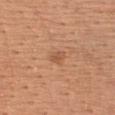Imaged during a routine full-body skin examination; the lesion was not biopsied and no histopathology is available.
About 2 mm across.
The total-body-photography lesion software estimated a footprint of about 2.5 mm², an outline eccentricity of about 0.75 (0 = round, 1 = elongated), and a symmetry-axis asymmetry near 0.25. And it measured a lesion color around L≈55 a*≈23 b*≈35 in CIELAB, roughly 8 lightness units darker than nearby skin, and a normalized border contrast of about 5.5. The analysis additionally found border irregularity of about 2 on a 0–10 scale, a within-lesion color-variation index near 1.5/10, and peripheral color asymmetry of about 0.5.
This is a white-light tile.
A lesion tile, about 15 mm wide, cut from a 3D total-body photograph.
A female subject, aged approximately 50.
From the chest.